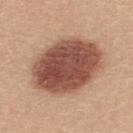follow-up: imaged on a skin check; not biopsied
acquisition: ~15 mm crop, total-body skin-cancer survey
patient: female, roughly 25 years of age
tile lighting: white-light
lesion size: ~9 mm (longest diameter)
location: the back